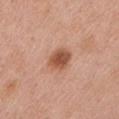Captured during whole-body skin photography for melanoma surveillance; the lesion was not biopsied. A male patient, aged around 55. Cropped from a whole-body photographic skin survey; the tile spans about 15 mm. Located on the left upper arm.